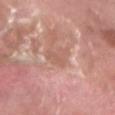Findings:
– illumination — white-light illumination
– image source — ~15 mm tile from a whole-body skin photo
– site — the left lower leg
– size — ~3 mm (longest diameter)
– subject — male, aged 38–42
– automated metrics — an area of roughly 4 mm², an outline eccentricity of about 0.8 (0 = round, 1 = elongated), and a symmetry-axis asymmetry near 0.45; border irregularity of about 4.5 on a 0–10 scale, internal color variation of about 1 on a 0–10 scale, and peripheral color asymmetry of about 0.5